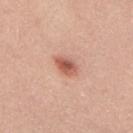The lesion was tiled from a total-body skin photograph and was not biopsied. The lesion is on the back. Captured under white-light illumination. A close-up tile cropped from a whole-body skin photograph, about 15 mm across. About 3 mm across. A male subject, approximately 25 years of age. The total-body-photography lesion software estimated a footprint of about 4.5 mm², a shape eccentricity near 0.75, and a symmetry-axis asymmetry near 0.2. The software also gave border irregularity of about 2 on a 0–10 scale and peripheral color asymmetry of about 1. The analysis additionally found a nevus-likeness score of about 95/100 and a detector confidence of about 100 out of 100 that the crop contains a lesion.